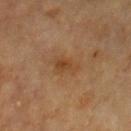The lesion was tiled from a total-body skin photograph and was not biopsied.
A 15 mm crop from a total-body photograph taken for skin-cancer surveillance.
The lesion is on the chest.
The total-body-photography lesion software estimated an area of roughly 6 mm² and a symmetry-axis asymmetry near 0.3. The analysis additionally found a lesion color around L≈38 a*≈18 b*≈31 in CIELAB and a normalized lesion–skin contrast near 6.
The tile uses cross-polarized illumination.
A male subject, in their mid- to late 80s or older.